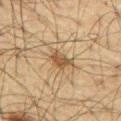– notes · imaged on a skin check; not biopsied
– image source · total-body-photography crop, ~15 mm field of view
– tile lighting · cross-polarized
– lesion diameter · ≈3.5 mm
– image-analysis metrics · an area of roughly 5.5 mm², an outline eccentricity of about 0.8 (0 = round, 1 = elongated), and a symmetry-axis asymmetry near 0.4
– site · the abdomen
– subject · male, roughly 65 years of age